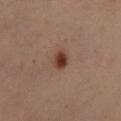• biopsy status — imaged on a skin check; not biopsied
• image source — ~15 mm tile from a whole-body skin photo
• patient — female, in their mid-50s
• illumination — cross-polarized illumination
• body site — the back
• size — about 2.5 mm